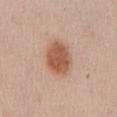Clinical impression:
This lesion was catalogued during total-body skin photography and was not selected for biopsy.
Acquisition and patient details:
About 5 mm across. On the back. A female patient, aged around 50. Imaged with white-light lighting. Cropped from a whole-body photographic skin survey; the tile spans about 15 mm.